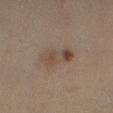Case summary:
- follow-up — imaged on a skin check; not biopsied
- automated metrics — an area of roughly 8.5 mm², an eccentricity of roughly 0.9, and two-axis asymmetry of about 0.2; a lesion color around L≈37 a*≈12 b*≈21 in CIELAB and a normalized border contrast of about 6.5; a classifier nevus-likeness of about 10/100 and a lesion-detection confidence of about 100/100
- acquisition — ~15 mm tile from a whole-body skin photo
- location — the left lower leg
- lighting — cross-polarized illumination
- subject — female, aged 38–42